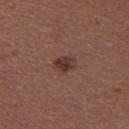Impression:
Recorded during total-body skin imaging; not selected for excision or biopsy.
Background:
A 15 mm crop from a total-body photograph taken for skin-cancer surveillance. Located on the upper back. Approximately 2.5 mm at its widest. Automated tile analysis of the lesion measured a footprint of about 4.5 mm², a shape eccentricity near 0.65, and two-axis asymmetry of about 0.2. The software also gave a mean CIELAB color near L≈36 a*≈20 b*≈23, a lesion–skin lightness drop of about 9, and a lesion-to-skin contrast of about 8 (normalized; higher = more distinct). It also reported a classifier nevus-likeness of about 90/100 and a detector confidence of about 100 out of 100 that the crop contains a lesion. A female patient, aged 23–27.